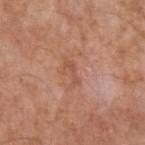| key | value |
|---|---|
| follow-up | no biopsy performed (imaged during a skin exam) |
| site | the left upper arm |
| lighting | white-light illumination |
| patient | male, aged 53–57 |
| automated lesion analysis | an outline eccentricity of about 0.95 (0 = round, 1 = elongated) and a shape-asymmetry score of about 0.5 (0 = symmetric); an average lesion color of about L≈53 a*≈25 b*≈32 (CIELAB), a lesion–skin lightness drop of about 7, and a normalized lesion–skin contrast near 5; border irregularity of about 7 on a 0–10 scale |
| image | ~15 mm crop, total-body skin-cancer survey |